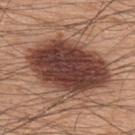Assessment: Recorded during total-body skin imaging; not selected for excision or biopsy. Acquisition and patient details: The tile uses white-light illumination. Measured at roughly 9 mm in maximum diameter. A region of skin cropped from a whole-body photographic capture, roughly 15 mm wide. A male patient, approximately 60 years of age. The lesion is on the back.